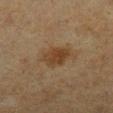Recorded during total-body skin imaging; not selected for excision or biopsy. Cropped from a whole-body photographic skin survey; the tile spans about 15 mm. The tile uses cross-polarized illumination. Longest diameter approximately 3.5 mm. The subject is a female aged approximately 55. From the left lower leg.